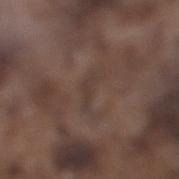Background: The lesion-visualizer software estimated an area of roughly 4 mm², a shape eccentricity near 0.9, and a symmetry-axis asymmetry near 0.6. The analysis additionally found a border-irregularity index near 6/10, a color-variation rating of about 1/10, and radial color variation of about 0.5. It also reported an automated nevus-likeness rating near 0 out of 100 and a detector confidence of about 50 out of 100 that the crop contains a lesion. The lesion is located on the right lower leg. A 15 mm crop from a total-body photograph taken for skin-cancer surveillance. A male subject aged 73 to 77.